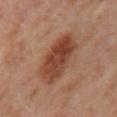{"biopsy_status": "not biopsied; imaged during a skin examination", "patient": {"sex": "female", "age_approx": 50}, "image": {"source": "total-body photography crop", "field_of_view_mm": 15}, "site": "right upper arm", "automated_metrics": {"cielab_L": 41, "cielab_a": 23, "cielab_b": 29, "vs_skin_darker_L": 11.0, "vs_skin_contrast_norm": 9.5, "border_irregularity_0_10": 2.0, "color_variation_0_10": 5.0, "peripheral_color_asymmetry": 1.5, "nevus_likeness_0_100": 95, "lesion_detection_confidence_0_100": 100}}